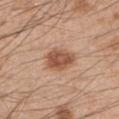notes: total-body-photography surveillance lesion; no biopsy | automated lesion analysis: a footprint of about 9 mm², an outline eccentricity of about 0.7 (0 = round, 1 = elongated), and a shape-asymmetry score of about 0.2 (0 = symmetric); an automated nevus-likeness rating near 95 out of 100 and a detector confidence of about 100 out of 100 that the crop contains a lesion | site: the left upper arm | lighting: white-light | patient: male, in their 50s | lesion size: ≈4 mm | imaging modality: total-body-photography crop, ~15 mm field of view.